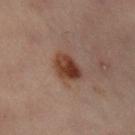The lesion was tiled from a total-body skin photograph and was not biopsied.
Cropped from a total-body skin-imaging series; the visible field is about 15 mm.
A female subject roughly 60 years of age.
Longest diameter approximately 4 mm.
An algorithmic analysis of the crop reported a lesion area of about 8 mm² and two-axis asymmetry of about 0.25. The analysis additionally found a lesion color around L≈34 a*≈18 b*≈24 in CIELAB, a lesion–skin lightness drop of about 10, and a normalized border contrast of about 10. The analysis additionally found a nevus-likeness score of about 100/100 and lesion-presence confidence of about 100/100.
This is a cross-polarized tile.
Located on the left leg.